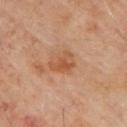{"biopsy_status": "not biopsied; imaged during a skin examination", "lesion_size": {"long_diameter_mm_approx": 3.0}, "site": "upper back", "lighting": "cross-polarized", "image": {"source": "total-body photography crop", "field_of_view_mm": 15}, "patient": {"sex": "male", "age_approx": 65}}